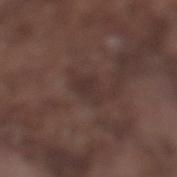<record>
  <biopsy_status>not biopsied; imaged during a skin examination</biopsy_status>
  <lighting>white-light</lighting>
  <lesion_size>
    <long_diameter_mm_approx>3.0</long_diameter_mm_approx>
  </lesion_size>
  <patient>
    <sex>male</sex>
    <age_approx>75</age_approx>
  </patient>
  <automated_metrics>
    <area_mm2_approx>4.0</area_mm2_approx>
    <eccentricity>0.75</eccentricity>
    <shape_asymmetry>0.35</shape_asymmetry>
    <cielab_L>31</cielab_L>
    <cielab_a>15</cielab_a>
    <cielab_b>17</cielab_b>
    <vs_skin_darker_L>6.0</vs_skin_darker_L>
    <vs_skin_contrast_norm>6.0</vs_skin_contrast_norm>
    <border_irregularity_0_10>4.0</border_irregularity_0_10>
  </automated_metrics>
  <image>
    <source>total-body photography crop</source>
    <field_of_view_mm>15</field_of_view_mm>
  </image>
  <site>left lower leg</site>
</record>